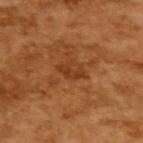No biopsy was performed on this lesion — it was imaged during a full skin examination and was not determined to be concerning. Automated image analysis of the tile measured an area of roughly 4 mm², a shape eccentricity near 0.85, and two-axis asymmetry of about 0.45. And it measured a mean CIELAB color near L≈37 a*≈25 b*≈37, about 7 CIELAB-L* units darker than the surrounding skin, and a lesion-to-skin contrast of about 6 (normalized; higher = more distinct). It also reported border irregularity of about 5.5 on a 0–10 scale and a within-lesion color-variation index near 0/10. And it measured an automated nevus-likeness rating near 0 out of 100. This is a cross-polarized tile. A region of skin cropped from a whole-body photographic capture, roughly 15 mm wide. The patient is a male aged around 65.